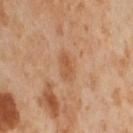biopsy status: catalogued during a skin exam; not biopsied.